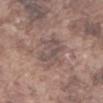Impression:
This lesion was catalogued during total-body skin photography and was not selected for biopsy.
Image and clinical context:
A male patient, about 75 years old. About 5 mm across. On the abdomen. Captured under white-light illumination. A 15 mm close-up tile from a total-body photography series done for melanoma screening. Automated tile analysis of the lesion measured a lesion area of about 12 mm² and an outline eccentricity of about 0.7 (0 = round, 1 = elongated). And it measured a lesion color around L≈50 a*≈15 b*≈19 in CIELAB, roughly 7 lightness units darker than nearby skin, and a lesion-to-skin contrast of about 6 (normalized; higher = more distinct). The software also gave a border-irregularity index near 4/10, internal color variation of about 3.5 on a 0–10 scale, and radial color variation of about 1.5. The software also gave a nevus-likeness score of about 0/100 and a lesion-detection confidence of about 55/100.